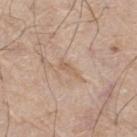Q: Was a biopsy performed?
A: total-body-photography surveillance lesion; no biopsy
Q: What kind of image is this?
A: total-body-photography crop, ~15 mm field of view
Q: What lighting was used for the tile?
A: white-light illumination
Q: How large is the lesion?
A: about 2.5 mm
Q: What is the anatomic site?
A: the right thigh
Q: What are the patient's age and sex?
A: male, aged approximately 70